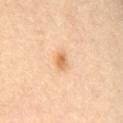Imaged during a routine full-body skin examination; the lesion was not biopsied and no histopathology is available. A 15 mm close-up extracted from a 3D total-body photography capture. Measured at roughly 2.5 mm in maximum diameter. A female subject about 65 years old. On the mid back.